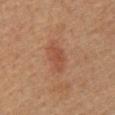This lesion was catalogued during total-body skin photography and was not selected for biopsy.
Automated tile analysis of the lesion measured a mean CIELAB color near L≈43 a*≈22 b*≈29 and a normalized border contrast of about 5.5. The software also gave border irregularity of about 3.5 on a 0–10 scale, internal color variation of about 1.5 on a 0–10 scale, and a peripheral color-asymmetry measure near 0.5. And it measured a classifier nevus-likeness of about 65/100.
The recorded lesion diameter is about 4 mm.
A region of skin cropped from a whole-body photographic capture, roughly 15 mm wide.
A male subject, approximately 60 years of age.
The lesion is on the mid back.
Imaged with cross-polarized lighting.